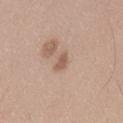Imaged during a routine full-body skin examination; the lesion was not biopsied and no histopathology is available.
This image is a 15 mm lesion crop taken from a total-body photograph.
About 2.5 mm across.
Automated tile analysis of the lesion measured border irregularity of about 3.5 on a 0–10 scale and internal color variation of about 1 on a 0–10 scale. The analysis additionally found a classifier nevus-likeness of about 0/100 and lesion-presence confidence of about 100/100.
From the left thigh.
A male patient about 35 years old.
The tile uses white-light illumination.